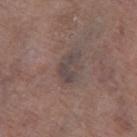| feature | finding |
|---|---|
| body site | the left thigh |
| lighting | white-light |
| size | ≈3.5 mm |
| patient | male, aged around 75 |
| image-analysis metrics | a border-irregularity rating of about 6/10, internal color variation of about 0.5 on a 0–10 scale, and radial color variation of about 0; a classifier nevus-likeness of about 0/100 |
| acquisition | total-body-photography crop, ~15 mm field of view |